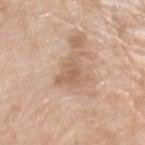Q: What are the patient's age and sex?
A: female, aged approximately 75
Q: What lighting was used for the tile?
A: white-light illumination
Q: What is the anatomic site?
A: the arm
Q: What is the imaging modality?
A: total-body-photography crop, ~15 mm field of view